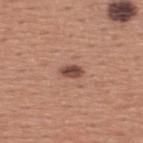Impression:
Captured during whole-body skin photography for melanoma surveillance; the lesion was not biopsied.
Background:
The lesion's longest dimension is about 2.5 mm. Located on the back. Captured under white-light illumination. A roughly 15 mm field-of-view crop from a total-body skin photograph. A male patient, aged around 45.